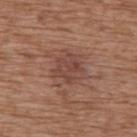{"biopsy_status": "not biopsied; imaged during a skin examination", "lighting": "white-light", "image": {"source": "total-body photography crop", "field_of_view_mm": 15}, "lesion_size": {"long_diameter_mm_approx": 3.5}, "site": "upper back", "patient": {"sex": "female", "age_approx": 75}}